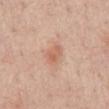Findings:
• biopsy status — catalogued during a skin exam; not biopsied
• tile lighting — white-light
• image — 15 mm crop, total-body photography
• automated lesion analysis — an average lesion color of about L≈62 a*≈24 b*≈31 (CIELAB), a lesion–skin lightness drop of about 8, and a normalized border contrast of about 5.5; an automated nevus-likeness rating near 30 out of 100 and a detector confidence of about 100 out of 100 that the crop contains a lesion
• patient — male, roughly 60 years of age
• lesion size — about 3 mm
• anatomic site — the abdomen